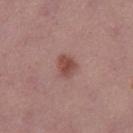Assessment: Imaged during a routine full-body skin examination; the lesion was not biopsied and no histopathology is available.